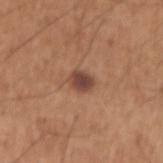Findings:
- biopsy status · total-body-photography surveillance lesion; no biopsy
- TBP lesion metrics · an area of roughly 4.5 mm² and a shape eccentricity near 0.6; a normalized lesion–skin contrast near 9.5; a border-irregularity rating of about 1.5/10 and a within-lesion color-variation index near 3/10; a nevus-likeness score of about 80/100
- lesion size · ~2.5 mm (longest diameter)
- location · the right upper arm
- subject · male, in their mid-60s
- imaging modality · total-body-photography crop, ~15 mm field of view